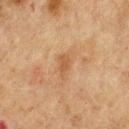workup = imaged on a skin check; not biopsied.